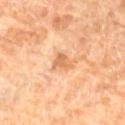Part of a total-body skin-imaging series; this lesion was reviewed on a skin check and was not flagged for biopsy. The subject is a female roughly 70 years of age. This image is a 15 mm lesion crop taken from a total-body photograph. The recorded lesion diameter is about 2.5 mm. This is a cross-polarized tile. The total-body-photography lesion software estimated roughly 10 lightness units darker than nearby skin and a normalized lesion–skin contrast near 6.5. And it measured a border-irregularity rating of about 3.5/10 and radial color variation of about 0.5. The analysis additionally found a nevus-likeness score of about 0/100 and lesion-presence confidence of about 100/100. Located on the left lower leg.